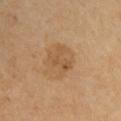  lighting: cross-polarized
  automated_metrics:
    area_mm2_approx: 4.0
    eccentricity: 0.8
    shape_asymmetry: 0.6
  patient:
    sex: male
    age_approx: 65
  lesion_size:
    long_diameter_mm_approx: 3.0
  image:
    source: total-body photography crop
    field_of_view_mm: 15
  site: right upper arm